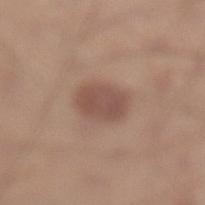Q: Is there a histopathology result?
A: total-body-photography surveillance lesion; no biopsy
Q: Illumination type?
A: white-light illumination
Q: Who is the patient?
A: male, about 30 years old
Q: What is the imaging modality?
A: 15 mm crop, total-body photography
Q: What did automated image analysis measure?
A: an eccentricity of roughly 0.7; a classifier nevus-likeness of about 80/100 and lesion-presence confidence of about 100/100
Q: What is the anatomic site?
A: the left lower leg